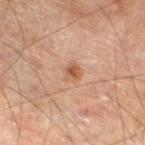Part of a total-body skin-imaging series; this lesion was reviewed on a skin check and was not flagged for biopsy. Captured under cross-polarized illumination. A 15 mm crop from a total-body photograph taken for skin-cancer surveillance. An algorithmic analysis of the crop reported a lesion area of about 3 mm², a shape eccentricity near 0.45, and a shape-asymmetry score of about 0.2 (0 = symmetric). And it measured border irregularity of about 2 on a 0–10 scale, a within-lesion color-variation index near 2.5/10, and radial color variation of about 1. The lesion is on the leg. A male subject aged approximately 65.